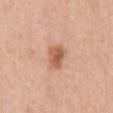A female patient, roughly 40 years of age. A 15 mm close-up tile from a total-body photography series done for melanoma screening. The tile uses white-light illumination. About 3 mm across. The lesion is located on the abdomen. An algorithmic analysis of the crop reported a lesion-to-skin contrast of about 8 (normalized; higher = more distinct). And it measured border irregularity of about 1.5 on a 0–10 scale, a color-variation rating of about 4/10, and peripheral color asymmetry of about 1.5. The software also gave a classifier nevus-likeness of about 85/100 and a lesion-detection confidence of about 100/100.